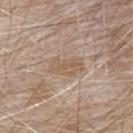follow-up: total-body-photography surveillance lesion; no biopsy
site: the upper back
tile lighting: white-light illumination
subject: male, roughly 80 years of age
image source: ~15 mm crop, total-body skin-cancer survey
diameter: about 4 mm